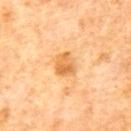Context: The lesion is on the mid back. This image is a 15 mm lesion crop taken from a total-body photograph. The lesion's longest dimension is about 2.5 mm. A male subject aged 63–67. Captured under cross-polarized illumination. Automated tile analysis of the lesion measured a footprint of about 5.5 mm², an eccentricity of roughly 0.6, and two-axis asymmetry of about 0.2.